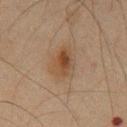The lesion was photographed on a routine skin check and not biopsied; there is no pathology result. Captured under cross-polarized illumination. A lesion tile, about 15 mm wide, cut from a 3D total-body photograph. The subject is a male in their mid-60s. Automated tile analysis of the lesion measured roughly 8 lightness units darker than nearby skin and a lesion-to-skin contrast of about 7.5 (normalized; higher = more distinct). And it measured lesion-presence confidence of about 100/100. The lesion is located on the chest.